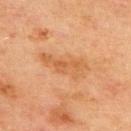No biopsy was performed on this lesion — it was imaged during a full skin examination and was not determined to be concerning. A male patient, about 70 years old. A 15 mm close-up tile from a total-body photography series done for melanoma screening. The lesion's longest dimension is about 3 mm. From the upper back.